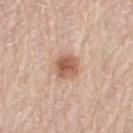<record>
<biopsy_status>not biopsied; imaged during a skin examination</biopsy_status>
<image>
  <source>total-body photography crop</source>
  <field_of_view_mm>15</field_of_view_mm>
</image>
<lighting>white-light</lighting>
<site>left thigh</site>
<patient>
  <sex>female</sex>
  <age_approx>70</age_approx>
</patient>
</record>